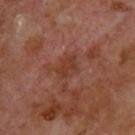Clinical impression:
Imaged during a routine full-body skin examination; the lesion was not biopsied and no histopathology is available.
Acquisition and patient details:
The total-body-photography lesion software estimated a lesion area of about 5.5 mm², an eccentricity of roughly 0.75, and a shape-asymmetry score of about 0.25 (0 = symmetric). The software also gave a lesion color around L≈37 a*≈24 b*≈28 in CIELAB and a lesion–skin lightness drop of about 6. And it measured an automated nevus-likeness rating near 0 out of 100 and lesion-presence confidence of about 100/100. Approximately 3 mm at its widest. This image is a 15 mm lesion crop taken from a total-body photograph. A male patient roughly 70 years of age. The lesion is on the upper back.